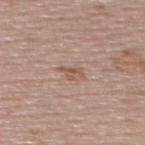Imaged during a routine full-body skin examination; the lesion was not biopsied and no histopathology is available. Measured at roughly 2.5 mm in maximum diameter. A roughly 15 mm field-of-view crop from a total-body skin photograph. Located on the upper back. A female subject, roughly 55 years of age. An algorithmic analysis of the crop reported a lesion area of about 3 mm² and a shape-asymmetry score of about 0.45 (0 = symmetric). It also reported about 8 CIELAB-L* units darker than the surrounding skin and a lesion-to-skin contrast of about 6 (normalized; higher = more distinct). The analysis additionally found border irregularity of about 5.5 on a 0–10 scale, internal color variation of about 0 on a 0–10 scale, and radial color variation of about 0.